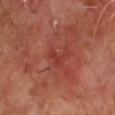| key | value |
|---|---|
| follow-up | no biopsy performed (imaged during a skin exam) |
| location | the chest |
| lesion size | about 3 mm |
| imaging modality | 15 mm crop, total-body photography |
| automated lesion analysis | a footprint of about 3 mm² and two-axis asymmetry of about 0.7; a mean CIELAB color near L≈37 a*≈32 b*≈27, roughly 6 lightness units darker than nearby skin, and a normalized border contrast of about 5; a peripheral color-asymmetry measure near 0; a nevus-likeness score of about 0/100 and a detector confidence of about 95 out of 100 that the crop contains a lesion |
| lighting | cross-polarized |
| patient | male, aged 63 to 67 |